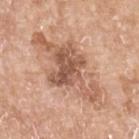Clinical impression: This lesion was catalogued during total-body skin photography and was not selected for biopsy. Acquisition and patient details: The lesion's longest dimension is about 10.5 mm. An algorithmic analysis of the crop reported border irregularity of about 7.5 on a 0–10 scale and radial color variation of about 1.5. The analysis additionally found an automated nevus-likeness rating near 15 out of 100. A female patient, approximately 75 years of age. A close-up tile cropped from a whole-body skin photograph, about 15 mm across. Imaged with white-light lighting. On the left forearm.